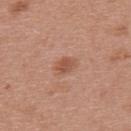Clinical impression: Recorded during total-body skin imaging; not selected for excision or biopsy. Image and clinical context: A female subject aged 38 to 42. This image is a 15 mm lesion crop taken from a total-body photograph. On the upper back.